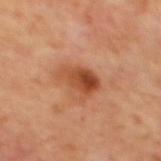Q: Is there a histopathology result?
A: catalogued during a skin exam; not biopsied
Q: What did automated image analysis measure?
A: a lesion area of about 8.5 mm² and two-axis asymmetry of about 0.3; a lesion color around L≈48 a*≈27 b*≈37 in CIELAB, about 12 CIELAB-L* units darker than the surrounding skin, and a lesion-to-skin contrast of about 9 (normalized; higher = more distinct); a classifier nevus-likeness of about 85/100 and a detector confidence of about 100 out of 100 that the crop contains a lesion
Q: Illumination type?
A: cross-polarized illumination
Q: Lesion size?
A: ≈3.5 mm
Q: What is the anatomic site?
A: the back
Q: Patient demographics?
A: roughly 65 years of age
Q: How was this image acquired?
A: 15 mm crop, total-body photography